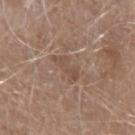Q: Is there a histopathology result?
A: total-body-photography surveillance lesion; no biopsy
Q: Lesion size?
A: about 3.5 mm
Q: Patient demographics?
A: male, roughly 75 years of age
Q: What lighting was used for the tile?
A: white-light
Q: What is the anatomic site?
A: the left forearm
Q: What is the imaging modality?
A: total-body-photography crop, ~15 mm field of view
Q: What did automated image analysis measure?
A: an area of roughly 5 mm², an outline eccentricity of about 0.9 (0 = round, 1 = elongated), and a shape-asymmetry score of about 0.45 (0 = symmetric); an average lesion color of about L≈49 a*≈17 b*≈26 (CIELAB), about 7 CIELAB-L* units darker than the surrounding skin, and a normalized border contrast of about 5; a detector confidence of about 80 out of 100 that the crop contains a lesion